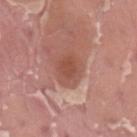Captured during whole-body skin photography for melanoma surveillance; the lesion was not biopsied. Measured at roughly 3.5 mm in maximum diameter. The lesion is on the left thigh. The tile uses white-light illumination. A 15 mm close-up extracted from a 3D total-body photography capture. A male patient, approximately 40 years of age. Automated tile analysis of the lesion measured an area of roughly 7.5 mm², an outline eccentricity of about 0.55 (0 = round, 1 = elongated), and a shape-asymmetry score of about 0.15 (0 = symmetric). It also reported a mean CIELAB color near L≈50 a*≈24 b*≈28, a lesion–skin lightness drop of about 9, and a lesion-to-skin contrast of about 6.5 (normalized; higher = more distinct).